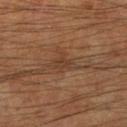follow-up: no biopsy performed (imaged during a skin exam)
tile lighting: cross-polarized
body site: the right lower leg
lesion size: ≈2.5 mm
TBP lesion metrics: a detector confidence of about 75 out of 100 that the crop contains a lesion
subject: male, aged 63 to 67
image: ~15 mm tile from a whole-body skin photo